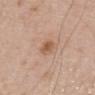Imaged during a routine full-body skin examination; the lesion was not biopsied and no histopathology is available.
The lesion is on the chest.
A male patient, aged 68–72.
Cropped from a whole-body photographic skin survey; the tile spans about 15 mm.
The recorded lesion diameter is about 2.5 mm.